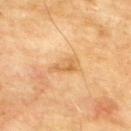workup = total-body-photography surveillance lesion; no biopsy
image source = 15 mm crop, total-body photography
location = the upper back
patient = male, in their mid- to late 70s